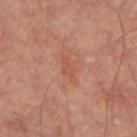Assessment: No biopsy was performed on this lesion — it was imaged during a full skin examination and was not determined to be concerning. Image and clinical context: The subject is a male roughly 70 years of age. Automated image analysis of the tile measured a footprint of about 3.5 mm² and an eccentricity of roughly 0.9. And it measured a lesion color around L≈51 a*≈24 b*≈32 in CIELAB, roughly 5 lightness units darker than nearby skin, and a lesion-to-skin contrast of about 5 (normalized; higher = more distinct). The software also gave a border-irregularity rating of about 3.5/10, a within-lesion color-variation index near 1/10, and a peripheral color-asymmetry measure near 0. The software also gave a classifier nevus-likeness of about 0/100 and lesion-presence confidence of about 100/100. Imaged with cross-polarized lighting. Cropped from a total-body skin-imaging series; the visible field is about 15 mm. On the leg.